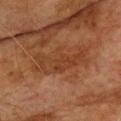The lesion was tiled from a total-body skin photograph and was not biopsied. The lesion is located on the upper back. Approximately 8 mm at its widest. A male patient, aged around 75. Cropped from a whole-body photographic skin survey; the tile spans about 15 mm.